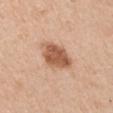Clinical impression: The lesion was photographed on a routine skin check and not biopsied; there is no pathology result. Context: A male subject, about 55 years old. About 4.5 mm across. Imaged with white-light lighting. From the arm. A 15 mm close-up extracted from a 3D total-body photography capture.